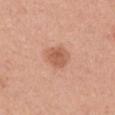Q: Was a biopsy performed?
A: no biopsy performed (imaged during a skin exam)
Q: Lesion location?
A: the left upper arm
Q: Who is the patient?
A: male, aged approximately 30
Q: How was this image acquired?
A: ~15 mm tile from a whole-body skin photo